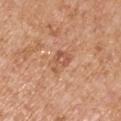{
  "biopsy_status": "not biopsied; imaged during a skin examination",
  "lesion_size": {
    "long_diameter_mm_approx": 3.0
  },
  "image": {
    "source": "total-body photography crop",
    "field_of_view_mm": 15
  },
  "automated_metrics": {
    "cielab_L": 56,
    "cielab_a": 24,
    "cielab_b": 34,
    "vs_skin_contrast_norm": 6.0,
    "border_irregularity_0_10": 5.0,
    "color_variation_0_10": 3.5,
    "nevus_likeness_0_100": 0,
    "lesion_detection_confidence_0_100": 100
  },
  "patient": {
    "sex": "male",
    "age_approx": 55
  },
  "lighting": "white-light",
  "site": "right upper arm"
}